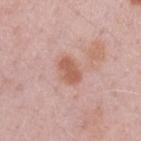The lesion was photographed on a routine skin check and not biopsied; there is no pathology result.
A region of skin cropped from a whole-body photographic capture, roughly 15 mm wide.
A female patient, aged 28–32.
Automated image analysis of the tile measured a lesion area of about 5.5 mm² and an outline eccentricity of about 0.8 (0 = round, 1 = elongated). The software also gave an average lesion color of about L≈58 a*≈24 b*≈29 (CIELAB), about 10 CIELAB-L* units darker than the surrounding skin, and a normalized border contrast of about 8. And it measured a border-irregularity rating of about 2.5/10, a color-variation rating of about 2/10, and peripheral color asymmetry of about 0.5. The analysis additionally found an automated nevus-likeness rating near 20 out of 100 and lesion-presence confidence of about 100/100.
Imaged with white-light lighting.
Located on the arm.
Measured at roughly 3 mm in maximum diameter.